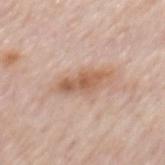* biopsy status: catalogued during a skin exam; not biopsied
* tile lighting: white-light
* patient: male, about 60 years old
* image source: ~15 mm crop, total-body skin-cancer survey
* lesion size: ≈5 mm
* anatomic site: the mid back